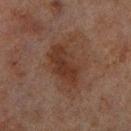Q: Was a biopsy performed?
A: total-body-photography surveillance lesion; no biopsy
Q: What lighting was used for the tile?
A: cross-polarized
Q: What is the lesion's diameter?
A: ~6 mm (longest diameter)
Q: Where on the body is the lesion?
A: the right lower leg
Q: Patient demographics?
A: male, approximately 70 years of age
Q: What is the imaging modality?
A: 15 mm crop, total-body photography